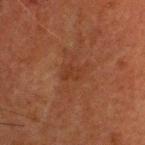Imaged during a routine full-body skin examination; the lesion was not biopsied and no histopathology is available. A close-up tile cropped from a whole-body skin photograph, about 15 mm across. The subject is a male aged around 50. The lesion-visualizer software estimated an area of roughly 4 mm², an outline eccentricity of about 0.7 (0 = round, 1 = elongated), and a shape-asymmetry score of about 0.35 (0 = symmetric). It also reported a mean CIELAB color near L≈28 a*≈20 b*≈27 and roughly 4 lightness units darker than nearby skin. This is a cross-polarized tile. The recorded lesion diameter is about 2.5 mm. On the head or neck.